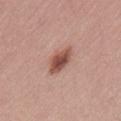Findings:
– biopsy status · no biopsy performed (imaged during a skin exam)
– patient · male, aged around 75
– automated lesion analysis · a footprint of about 7 mm², a shape eccentricity near 0.75, and two-axis asymmetry of about 0.2; a border-irregularity rating of about 2/10 and a within-lesion color-variation index near 4.5/10; an automated nevus-likeness rating near 95 out of 100
– lesion size · ≈4 mm
– anatomic site · the lower back
– imaging modality · ~15 mm tile from a whole-body skin photo
– illumination · white-light